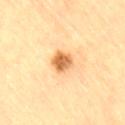workup: total-body-photography surveillance lesion; no biopsy